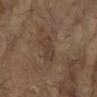A 15 mm close-up extracted from a 3D total-body photography capture.
Located on the left arm.
A female subject about 80 years old.